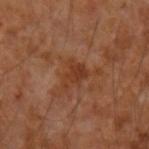Q: Where on the body is the lesion?
A: the left forearm
Q: What are the patient's age and sex?
A: male, aged around 55
Q: What kind of image is this?
A: 15 mm crop, total-body photography